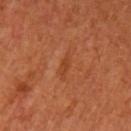Q: Was this lesion biopsied?
A: catalogued during a skin exam; not biopsied
Q: What is the lesion's diameter?
A: about 2.5 mm
Q: What kind of image is this?
A: total-body-photography crop, ~15 mm field of view
Q: What is the anatomic site?
A: the left upper arm
Q: What did automated image analysis measure?
A: a lesion area of about 2.5 mm²; a nevus-likeness score of about 0/100 and a lesion-detection confidence of about 100/100
Q: What are the patient's age and sex?
A: female, approximately 65 years of age